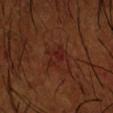follow-up: imaged on a skin check; not biopsied | subject: male, in their mid-60s | imaging modality: 15 mm crop, total-body photography | location: the arm | lighting: cross-polarized.